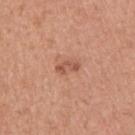Q: Was a biopsy performed?
A: total-body-photography surveillance lesion; no biopsy
Q: Patient demographics?
A: male, approximately 50 years of age
Q: What is the imaging modality?
A: ~15 mm tile from a whole-body skin photo
Q: Lesion location?
A: the upper back
Q: How was the tile lit?
A: white-light
Q: What did automated image analysis measure?
A: a within-lesion color-variation index near 0/10; a nevus-likeness score of about 15/100 and a lesion-detection confidence of about 100/100
Q: What is the lesion's diameter?
A: ~3 mm (longest diameter)